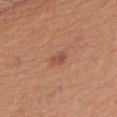The lesion was photographed on a routine skin check and not biopsied; there is no pathology result.
A male patient aged 38 to 42.
From the arm.
The recorded lesion diameter is about 2.5 mm.
Cropped from a total-body skin-imaging series; the visible field is about 15 mm.